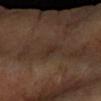This lesion was catalogued during total-body skin photography and was not selected for biopsy.
Longest diameter approximately 3 mm.
The lesion is located on the left forearm.
This is a cross-polarized tile.
The patient is a female roughly 60 years of age.
A 15 mm close-up tile from a total-body photography series done for melanoma screening.